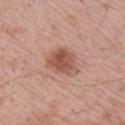Q: Is there a histopathology result?
A: catalogued during a skin exam; not biopsied
Q: Where on the body is the lesion?
A: the arm
Q: What is the imaging modality?
A: total-body-photography crop, ~15 mm field of view
Q: Lesion size?
A: ≈4.5 mm
Q: Patient demographics?
A: male, aged around 65